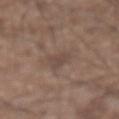Recorded during total-body skin imaging; not selected for excision or biopsy. A male patient, about 75 years old. A 15 mm crop from a total-body photograph taken for skin-cancer surveillance. The lesion is on the abdomen.